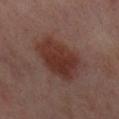follow-up — imaged on a skin check; not biopsied
imaging modality — ~15 mm crop, total-body skin-cancer survey
subject — female, in their 50s
location — the left thigh
lighting — cross-polarized illumination